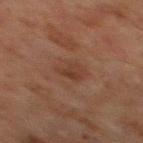Assessment:
Part of a total-body skin-imaging series; this lesion was reviewed on a skin check and was not flagged for biopsy.
Clinical summary:
This image is a 15 mm lesion crop taken from a total-body photograph. Located on the chest. A male subject, aged 63–67.